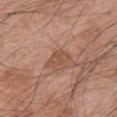Part of a total-body skin-imaging series; this lesion was reviewed on a skin check and was not flagged for biopsy.
The tile uses white-light illumination.
The lesion's longest dimension is about 3 mm.
The lesion is on the chest.
A male subject aged 68–72.
A close-up tile cropped from a whole-body skin photograph, about 15 mm across.
Automated tile analysis of the lesion measured a lesion–skin lightness drop of about 8.